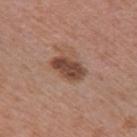<case>
  <biopsy_status>not biopsied; imaged during a skin examination</biopsy_status>
  <lighting>white-light</lighting>
  <site>right upper arm</site>
  <lesion_size>
    <long_diameter_mm_approx>4.0</long_diameter_mm_approx>
  </lesion_size>
  <automated_metrics>
    <cielab_L>45</cielab_L>
    <cielab_a>20</cielab_a>
    <cielab_b>27</cielab_b>
    <vs_skin_darker_L>13.0</vs_skin_darker_L>
    <border_irregularity_0_10>2.0</border_irregularity_0_10>
    <color_variation_0_10>3.5</color_variation_0_10>
    <peripheral_color_asymmetry>1.5</peripheral_color_asymmetry>
  </automated_metrics>
  <patient>
    <sex>male</sex>
    <age_approx>70</age_approx>
  </patient>
  <image>
    <source>total-body photography crop</source>
    <field_of_view_mm>15</field_of_view_mm>
  </image>
</case>